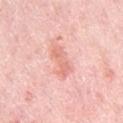Captured during whole-body skin photography for melanoma surveillance; the lesion was not biopsied. Approximately 4 mm at its widest. Captured under white-light illumination. On the left upper arm. A female subject, in their mid- to late 60s. A region of skin cropped from a whole-body photographic capture, roughly 15 mm wide. An algorithmic analysis of the crop reported an area of roughly 5.5 mm². The analysis additionally found a border-irregularity rating of about 4/10 and a peripheral color-asymmetry measure near 0.5.